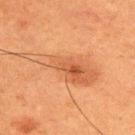Case summary:
• biopsy status — catalogued during a skin exam; not biopsied
• image — ~15 mm crop, total-body skin-cancer survey
• tile lighting — cross-polarized illumination
• patient — male, aged 48–52
• site — the back